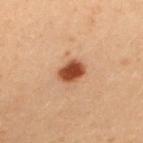Findings:
* anatomic site: the upper back
* diameter: ≈3 mm
* acquisition: total-body-photography crop, ~15 mm field of view
* illumination: cross-polarized illumination
* patient: female, approximately 30 years of age
* TBP lesion metrics: an area of roughly 5.5 mm², an outline eccentricity of about 0.75 (0 = round, 1 = elongated), and a shape-asymmetry score of about 0.2 (0 = symmetric); a border-irregularity rating of about 2/10, internal color variation of about 2 on a 0–10 scale, and radial color variation of about 0.5; a nevus-likeness score of about 100/100